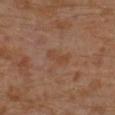{"biopsy_status": "not biopsied; imaged during a skin examination", "automated_metrics": {"area_mm2_approx": 3.0, "eccentricity": 0.9, "shape_asymmetry": 0.4, "border_irregularity_0_10": 4.5, "color_variation_0_10": 0.0, "peripheral_color_asymmetry": 0.0}, "site": "left leg", "patient": {"sex": "male", "age_approx": 30}, "lighting": "cross-polarized", "image": {"source": "total-body photography crop", "field_of_view_mm": 15}}